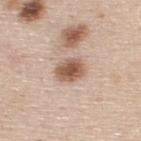The lesion was tiled from a total-body skin photograph and was not biopsied. Approximately 3.5 mm at its widest. A 15 mm close-up extracted from a 3D total-body photography capture. A male subject, roughly 45 years of age. Imaged with white-light lighting. Located on the upper back.